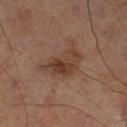No biopsy was performed on this lesion — it was imaged during a full skin examination and was not determined to be concerning. Automated tile analysis of the lesion measured an area of roughly 13 mm², an eccentricity of roughly 0.85, and a symmetry-axis asymmetry near 0.45. The software also gave a lesion color around L≈31 a*≈15 b*≈22 in CIELAB, a lesion–skin lightness drop of about 7, and a normalized border contrast of about 7. The analysis additionally found border irregularity of about 5.5 on a 0–10 scale, internal color variation of about 5 on a 0–10 scale, and peripheral color asymmetry of about 2. And it measured an automated nevus-likeness rating near 15 out of 100 and lesion-presence confidence of about 100/100. A male subject, aged 68 to 72. This is a cross-polarized tile. From the leg. A 15 mm close-up extracted from a 3D total-body photography capture. The recorded lesion diameter is about 6 mm.